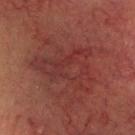Clinical impression:
The lesion was photographed on a routine skin check and not biopsied; there is no pathology result.